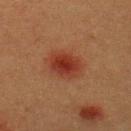<tbp_lesion>
  <biopsy_status>not biopsied; imaged during a skin examination</biopsy_status>
  <image>
    <source>total-body photography crop</source>
    <field_of_view_mm>15</field_of_view_mm>
  </image>
  <lighting>cross-polarized</lighting>
  <automated_metrics>
    <area_mm2_approx>9.5</area_mm2_approx>
    <eccentricity>0.7</eccentricity>
    <shape_asymmetry>0.15</shape_asymmetry>
    <cielab_L>30</cielab_L>
    <cielab_a>25</cielab_a>
    <cielab_b>27</cielab_b>
    <vs_skin_contrast_norm>8.5</vs_skin_contrast_norm>
  </automated_metrics>
  <site>upper back</site>
  <patient>
    <sex>male</sex>
    <age_approx>40</age_approx>
  </patient>
  <lesion_size>
    <long_diameter_mm_approx>4.0</long_diameter_mm_approx>
  </lesion_size>
</tbp_lesion>